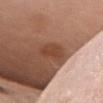Part of a total-body skin-imaging series; this lesion was reviewed on a skin check and was not flagged for biopsy. On the chest. A female subject, aged 48–52. A 15 mm crop from a total-body photograph taken for skin-cancer surveillance.